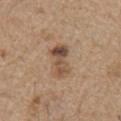Captured during whole-body skin photography for melanoma surveillance; the lesion was not biopsied. Imaged with white-light lighting. The lesion is located on the chest. About 4.5 mm across. A region of skin cropped from a whole-body photographic capture, roughly 15 mm wide. A male subject, approximately 70 years of age. An algorithmic analysis of the crop reported a lesion area of about 8.5 mm², an outline eccentricity of about 0.85 (0 = round, 1 = elongated), and a symmetry-axis asymmetry near 0.3. And it measured border irregularity of about 3.5 on a 0–10 scale, a within-lesion color-variation index near 8.5/10, and radial color variation of about 3.